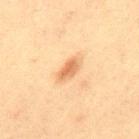Captured during whole-body skin photography for melanoma surveillance; the lesion was not biopsied. Longest diameter approximately 3 mm. A lesion tile, about 15 mm wide, cut from a 3D total-body photograph. The patient is a male approximately 60 years of age. On the back.